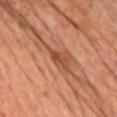Notes:
• workup — no biopsy performed (imaged during a skin exam)
• site — the chest
• size — ≈2.5 mm
• image — 15 mm crop, total-body photography
• image-analysis metrics — a footprint of about 3 mm², a shape eccentricity near 0.9, and two-axis asymmetry of about 0.3; a lesion color around L≈49 a*≈28 b*≈36 in CIELAB, roughly 10 lightness units darker than nearby skin, and a normalized lesion–skin contrast near 7.5; border irregularity of about 3.5 on a 0–10 scale, a color-variation rating of about 0.5/10, and peripheral color asymmetry of about 0; a classifier nevus-likeness of about 0/100 and lesion-presence confidence of about 95/100
• patient — female, aged approximately 60